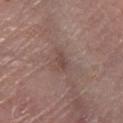{"lighting": "white-light", "image": {"source": "total-body photography crop", "field_of_view_mm": 15}, "lesion_size": {"long_diameter_mm_approx": 2.5}, "site": "left lower leg", "patient": {"sex": "male", "age_approx": 80}, "automated_metrics": {"area_mm2_approx": 3.0, "eccentricity": 0.85, "shape_asymmetry": 0.4, "cielab_L": 45, "cielab_a": 18, "cielab_b": 21, "vs_skin_darker_L": 7.0, "border_irregularity_0_10": 4.5, "peripheral_color_asymmetry": 0.5, "nevus_likeness_0_100": 0, "lesion_detection_confidence_0_100": 100}}